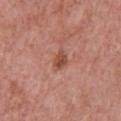This lesion was catalogued during total-body skin photography and was not selected for biopsy. A lesion tile, about 15 mm wide, cut from a 3D total-body photograph. On the chest. The patient is a male aged around 50.